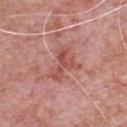{"biopsy_status": "not biopsied; imaged during a skin examination", "automated_metrics": {"cielab_L": 52, "cielab_a": 28, "cielab_b": 26, "vs_skin_darker_L": 9.0, "vs_skin_contrast_norm": 6.5, "border_irregularity_0_10": 6.5, "peripheral_color_asymmetry": 1.5, "nevus_likeness_0_100": 0, "lesion_detection_confidence_0_100": 100}, "patient": {"sex": "male", "age_approx": 80}, "site": "front of the torso", "image": {"source": "total-body photography crop", "field_of_view_mm": 15}, "lesion_size": {"long_diameter_mm_approx": 4.0}}